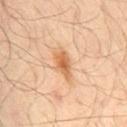Assessment:
The lesion was tiled from a total-body skin photograph and was not biopsied.
Background:
The lesion's longest dimension is about 3.5 mm. The tile uses cross-polarized illumination. A male subject, about 65 years old. Cropped from a total-body skin-imaging series; the visible field is about 15 mm. On the mid back.